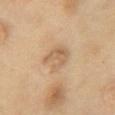biopsy_status: not biopsied; imaged during a skin examination
site: chest
patient:
  sex: female
  age_approx: 55
lighting: cross-polarized
image:
  source: total-body photography crop
  field_of_view_mm: 15
automated_metrics:
  area_mm2_approx: 7.0
  eccentricity: 0.6
  shape_asymmetry: 0.3
  color_variation_0_10: 3.5
  peripheral_color_asymmetry: 1.0